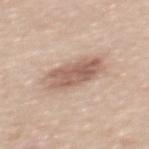biopsy status: imaged on a skin check; not biopsied | acquisition: ~15 mm crop, total-body skin-cancer survey | anatomic site: the mid back | patient: male, aged 48 to 52 | automated metrics: a lesion area of about 15 mm², an outline eccentricity of about 0.85 (0 = round, 1 = elongated), and a symmetry-axis asymmetry near 0.15; a lesion color around L≈60 a*≈18 b*≈26 in CIELAB, a lesion–skin lightness drop of about 12, and a normalized border contrast of about 7.5.